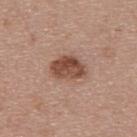  biopsy_status: not biopsied; imaged during a skin examination
  site: upper back
  patient:
    sex: female
    age_approx: 45
  image:
    source: total-body photography crop
    field_of_view_mm: 15